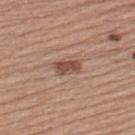Located on the upper back.
The total-body-photography lesion software estimated an area of roughly 4.5 mm² and a symmetry-axis asymmetry near 0.25. And it measured a lesion color around L≈49 a*≈20 b*≈28 in CIELAB, a lesion–skin lightness drop of about 12, and a normalized lesion–skin contrast near 8.5. The analysis additionally found a nevus-likeness score of about 75/100 and a detector confidence of about 100 out of 100 that the crop contains a lesion.
The tile uses white-light illumination.
Approximately 3 mm at its widest.
A close-up tile cropped from a whole-body skin photograph, about 15 mm across.
A male patient in their mid-50s.